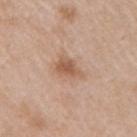{
  "biopsy_status": "not biopsied; imaged during a skin examination",
  "lesion_size": {
    "long_diameter_mm_approx": 3.5
  },
  "lighting": "white-light",
  "site": "arm",
  "image": {
    "source": "total-body photography crop",
    "field_of_view_mm": 15
  },
  "patient": {
    "sex": "female",
    "age_approx": 40
  }
}